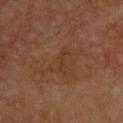Q: Was this lesion biopsied?
A: total-body-photography surveillance lesion; no biopsy
Q: Illumination type?
A: cross-polarized
Q: Lesion location?
A: the upper back
Q: What did automated image analysis measure?
A: a footprint of about 2 mm² and a shape eccentricity near 0.95; a lesion-detection confidence of about 100/100
Q: What are the patient's age and sex?
A: male, aged approximately 60
Q: What kind of image is this?
A: ~15 mm crop, total-body skin-cancer survey